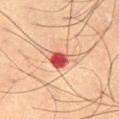<case>
<biopsy_status>not biopsied; imaged during a skin examination</biopsy_status>
<lighting>cross-polarized</lighting>
<automated_metrics>
  <area_mm2_approx>5.0</area_mm2_approx>
  <eccentricity>0.55</eccentricity>
  <shape_asymmetry>0.15</shape_asymmetry>
  <cielab_L>44</cielab_L>
  <cielab_a>33</cielab_a>
  <cielab_b>27</cielab_b>
  <vs_skin_darker_L>16.0</vs_skin_darker_L>
  <vs_skin_contrast_norm>12.0</vs_skin_contrast_norm>
  <border_irregularity_0_10>1.0</border_irregularity_0_10>
  <color_variation_0_10>3.5</color_variation_0_10>
  <peripheral_color_asymmetry>1.0</peripheral_color_asymmetry>
  <nevus_likeness_0_100>0</nevus_likeness_0_100>
</automated_metrics>
<site>leg</site>
<image>
  <source>total-body photography crop</source>
  <field_of_view_mm>15</field_of_view_mm>
</image>
<patient>
  <sex>male</sex>
  <age_approx>65</age_approx>
</patient>
<lesion_size>
  <long_diameter_mm_approx>2.5</long_diameter_mm_approx>
</lesion_size>
</case>